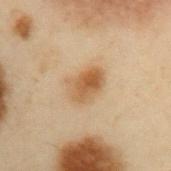No biopsy was performed on this lesion — it was imaged during a full skin examination and was not determined to be concerning. Approximately 4 mm at its widest. Cropped from a whole-body photographic skin survey; the tile spans about 15 mm. A male patient aged 53–57. The lesion is on the left upper arm. Imaged with cross-polarized lighting.